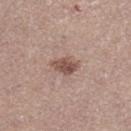Findings:
– site · the right lower leg
– size · ~3 mm (longest diameter)
– subject · female, about 35 years old
– image source · 15 mm crop, total-body photography